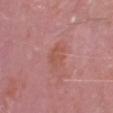Clinical impression: No biopsy was performed on this lesion — it was imaged during a full skin examination and was not determined to be concerning.